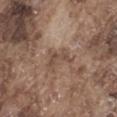| key | value |
|---|---|
| biopsy status | catalogued during a skin exam; not biopsied |
| automated lesion analysis | a lesion color around L≈47 a*≈17 b*≈26 in CIELAB and a normalized lesion–skin contrast near 6; border irregularity of about 6.5 on a 0–10 scale, a color-variation rating of about 2/10, and peripheral color asymmetry of about 1 |
| location | the left thigh |
| patient | male, approximately 75 years of age |
| image source | ~15 mm tile from a whole-body skin photo |
| illumination | white-light illumination |
| size | ~3.5 mm (longest diameter) |